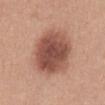workup — total-body-photography surveillance lesion; no biopsy | location — the front of the torso | image-analysis metrics — an eccentricity of roughly 0.4 and a shape-asymmetry score of about 0.1 (0 = symmetric); a mean CIELAB color near L≈50 a*≈23 b*≈27, a lesion–skin lightness drop of about 15, and a lesion-to-skin contrast of about 10 (normalized; higher = more distinct); border irregularity of about 1 on a 0–10 scale, a color-variation rating of about 5/10, and radial color variation of about 1.5; a detector confidence of about 100 out of 100 that the crop contains a lesion | tile lighting — white-light illumination | acquisition — total-body-photography crop, ~15 mm field of view | size — about 6.5 mm | subject — female, aged 38 to 42.